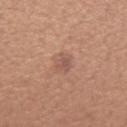Case summary:
• biopsy status: no biopsy performed (imaged during a skin exam)
• lesion size: about 2.5 mm
• patient: female, about 40 years old
• location: the arm
• image: ~15 mm tile from a whole-body skin photo
• lighting: white-light illumination